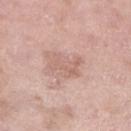follow-up = catalogued during a skin exam; not biopsied
lighting = white-light illumination
size = about 4.5 mm
image source = 15 mm crop, total-body photography
site = the left lower leg
subject = female, aged approximately 70